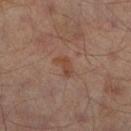No biopsy was performed on this lesion — it was imaged during a full skin examination and was not determined to be concerning.
Located on the right lower leg.
A roughly 15 mm field-of-view crop from a total-body skin photograph.
A male patient, about 65 years old.